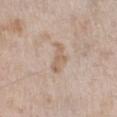No biopsy was performed on this lesion — it was imaged during a full skin examination and was not determined to be concerning.
A roughly 15 mm field-of-view crop from a total-body skin photograph.
The tile uses white-light illumination.
On the right lower leg.
A male subject, aged 58 to 62.